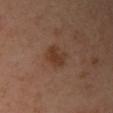Q: How was the tile lit?
A: cross-polarized illumination
Q: How was this image acquired?
A: total-body-photography crop, ~15 mm field of view
Q: Lesion location?
A: the left arm
Q: How large is the lesion?
A: about 3 mm
Q: Who is the patient?
A: female, approximately 40 years of age